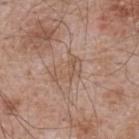No biopsy was performed on this lesion — it was imaged during a full skin examination and was not determined to be concerning.
A male patient, roughly 65 years of age.
A close-up tile cropped from a whole-body skin photograph, about 15 mm across.
Longest diameter approximately 4 mm.
The lesion is located on the upper back.